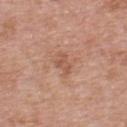Q: Was a biopsy performed?
A: imaged on a skin check; not biopsied
Q: What did automated image analysis measure?
A: an average lesion color of about L≈55 a*≈22 b*≈30 (CIELAB), a lesion–skin lightness drop of about 8, and a normalized border contrast of about 5.5; a border-irregularity index near 3.5/10 and internal color variation of about 2.5 on a 0–10 scale; a nevus-likeness score of about 0/100 and lesion-presence confidence of about 100/100
Q: What is the imaging modality?
A: ~15 mm tile from a whole-body skin photo
Q: What lighting was used for the tile?
A: white-light illumination
Q: Where on the body is the lesion?
A: the chest
Q: What are the patient's age and sex?
A: male, aged approximately 70